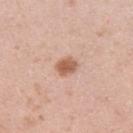Case summary:
- image · ~15 mm tile from a whole-body skin photo
- site · the left upper arm
- patient · male, aged 38 to 42
- size · ~2.5 mm (longest diameter)
- illumination · white-light illumination